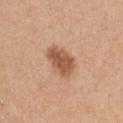Recorded during total-body skin imaging; not selected for excision or biopsy. A close-up tile cropped from a whole-body skin photograph, about 15 mm across. The subject is a female aged 43 to 47. Located on the chest. The tile uses white-light illumination. Measured at roughly 4.5 mm in maximum diameter.